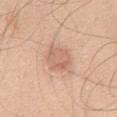biopsy_status: not biopsied; imaged during a skin examination
site: chest
patient:
  sex: male
  age_approx: 45
image:
  source: total-body photography crop
  field_of_view_mm: 15